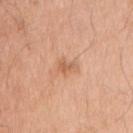biopsy_status: not biopsied; imaged during a skin examination
lighting: white-light
image:
  source: total-body photography crop
  field_of_view_mm: 15
patient:
  sex: male
  age_approx: 70
lesion_size:
  long_diameter_mm_approx: 2.5
site: right upper arm